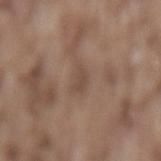Case summary:
- notes · catalogued during a skin exam; not biopsied
- TBP lesion metrics · a mean CIELAB color near L≈46 a*≈16 b*≈25, roughly 7 lightness units darker than nearby skin, and a normalized border contrast of about 5.5; a border-irregularity index near 3/10 and a color-variation rating of about 1/10; a classifier nevus-likeness of about 0/100 and lesion-presence confidence of about 95/100
- imaging modality · 15 mm crop, total-body photography
- size · ≈2.5 mm
- site · the lower back
- subject · male, aged approximately 75
- tile lighting · white-light illumination